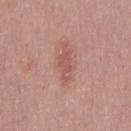{
  "biopsy_status": "not biopsied; imaged during a skin examination",
  "automated_metrics": {
    "area_mm2_approx": 5.0,
    "eccentricity": 0.9,
    "cielab_L": 55,
    "cielab_a": 26,
    "cielab_b": 25,
    "vs_skin_contrast_norm": 5.5,
    "nevus_likeness_0_100": 10,
    "lesion_detection_confidence_0_100": 100
  },
  "site": "chest",
  "patient": {
    "sex": "male",
    "age_approx": 45
  },
  "lesion_size": {
    "long_diameter_mm_approx": 3.5
  },
  "image": {
    "source": "total-body photography crop",
    "field_of_view_mm": 15
  }
}